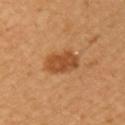{"automated_metrics": {"area_mm2_approx": 9.0, "eccentricity": 0.6, "shape_asymmetry": 0.15, "vs_skin_darker_L": 11.0, "vs_skin_contrast_norm": 8.0}, "lighting": "cross-polarized", "lesion_size": {"long_diameter_mm_approx": 3.5}, "image": {"source": "total-body photography crop", "field_of_view_mm": 15}, "patient": {"sex": "female", "age_approx": 35}, "site": "left upper arm"}